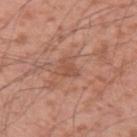notes = no biopsy performed (imaged during a skin exam) | image-analysis metrics = a lesion–skin lightness drop of about 7 and a normalized lesion–skin contrast near 5 | subject = male, aged around 40 | site = the arm | diameter = about 2.5 mm | tile lighting = white-light | image = ~15 mm tile from a whole-body skin photo.